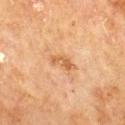{
  "biopsy_status": "not biopsied; imaged during a skin examination",
  "patient": {
    "sex": "male",
    "age_approx": 70
  },
  "image": {
    "source": "total-body photography crop",
    "field_of_view_mm": 15
  },
  "site": "mid back"
}